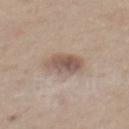Findings:
* subject · female, aged 38–42
* body site · the mid back
* imaging modality · ~15 mm tile from a whole-body skin photo
* lesion size · ≈3.5 mm
* lighting · white-light illumination
* automated metrics · an area of roughly 8.5 mm², a shape eccentricity near 0.6, and a shape-asymmetry score of about 0.2 (0 = symmetric); a mean CIELAB color near L≈54 a*≈16 b*≈25, roughly 11 lightness units darker than nearby skin, and a normalized lesion–skin contrast near 7.5